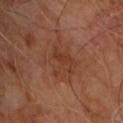workup=no biopsy performed (imaged during a skin exam)
anatomic site=the back
patient=male, roughly 60 years of age
size=~4 mm (longest diameter)
image source=~15 mm tile from a whole-body skin photo